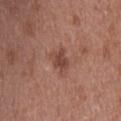Imaged during a routine full-body skin examination; the lesion was not biopsied and no histopathology is available. Automated tile analysis of the lesion measured a footprint of about 4.5 mm². The analysis additionally found a border-irregularity rating of about 3/10, a within-lesion color-variation index near 2/10, and a peripheral color-asymmetry measure near 0.5. Imaged with white-light lighting. A female patient aged approximately 40. From the head or neck. A roughly 15 mm field-of-view crop from a total-body skin photograph.